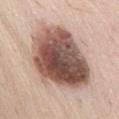notes = no biopsy performed (imaged during a skin exam); image = ~15 mm crop, total-body skin-cancer survey; anatomic site = the back; subject = male, aged around 70.